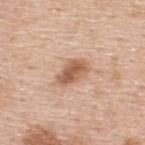image-analysis metrics: an eccentricity of roughly 0.8 and a shape-asymmetry score of about 0.2 (0 = symmetric); a mean CIELAB color near L≈59 a*≈21 b*≈32, roughly 13 lightness units darker than nearby skin, and a normalized lesion–skin contrast near 8.5; a border-irregularity index near 2/10, a within-lesion color-variation index near 4.5/10, and peripheral color asymmetry of about 1.5; an automated nevus-likeness rating near 85 out of 100 and a lesion-detection confidence of about 100/100
diameter: ≈4 mm
patient: male, aged approximately 50
acquisition: 15 mm crop, total-body photography
illumination: white-light
site: the back